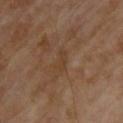notes: catalogued during a skin exam; not biopsied.